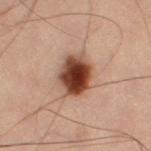| field | value |
|---|---|
| workup | imaged on a skin check; not biopsied |
| body site | the leg |
| imaging modality | ~15 mm crop, total-body skin-cancer survey |
| subject | male, approximately 60 years of age |